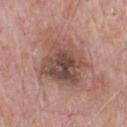The lesion was photographed on a routine skin check and not biopsied; there is no pathology result. A lesion tile, about 15 mm wide, cut from a 3D total-body photograph. Approximately 6.5 mm at its widest. A male subject approximately 70 years of age. The lesion is located on the chest. Imaged with white-light lighting.